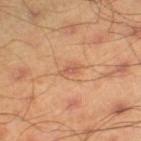Findings:
- follow-up — imaged on a skin check; not biopsied
- diameter — about 2.5 mm
- site — the left thigh
- lighting — cross-polarized
- automated metrics — a border-irregularity index near 3/10, a color-variation rating of about 0.5/10, and peripheral color asymmetry of about 0
- image — total-body-photography crop, ~15 mm field of view
- subject — male, approximately 45 years of age